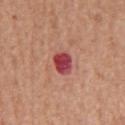Assessment: This lesion was catalogued during total-body skin photography and was not selected for biopsy. Image and clinical context: Cropped from a total-body skin-imaging series; the visible field is about 15 mm. Located on the chest. The subject is a male approximately 65 years of age. About 3 mm across. This is a white-light tile.